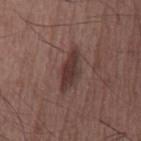Assessment: No biopsy was performed on this lesion — it was imaged during a full skin examination and was not determined to be concerning. Acquisition and patient details: The recorded lesion diameter is about 5 mm. The lesion is located on the chest. The total-body-photography lesion software estimated a lesion area of about 8.5 mm². And it measured border irregularity of about 3 on a 0–10 scale, internal color variation of about 2.5 on a 0–10 scale, and peripheral color asymmetry of about 1. The analysis additionally found an automated nevus-likeness rating near 45 out of 100. A male subject, about 60 years old. A region of skin cropped from a whole-body photographic capture, roughly 15 mm wide.